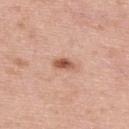Findings:
– image · ~15 mm tile from a whole-body skin photo
– patient · male, aged around 40
– site · the upper back